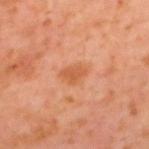notes — imaged on a skin check; not biopsied
patient — male, aged approximately 60
lesion diameter — ~3.5 mm (longest diameter)
acquisition — 15 mm crop, total-body photography
tile lighting — cross-polarized
location — the mid back